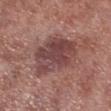Findings:
* follow-up · catalogued during a skin exam; not biopsied
* body site · the right lower leg
* tile lighting · white-light illumination
* patient · male, in their mid-50s
* acquisition · ~15 mm tile from a whole-body skin photo
* lesion size · ~6.5 mm (longest diameter)
* image-analysis metrics · a lesion color around L≈44 a*≈22 b*≈20 in CIELAB, a lesion–skin lightness drop of about 11, and a normalized border contrast of about 8.5; a within-lesion color-variation index near 5/10 and peripheral color asymmetry of about 1.5